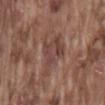patient = male, aged around 75; site = the lower back; image source = 15 mm crop, total-body photography.